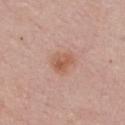Recorded during total-body skin imaging; not selected for excision or biopsy.
Approximately 3 mm at its widest.
A region of skin cropped from a whole-body photographic capture, roughly 15 mm wide.
A male subject, aged 58 to 62.
The lesion is located on the chest.
The lesion-visualizer software estimated a border-irregularity index near 2.5/10, internal color variation of about 4 on a 0–10 scale, and peripheral color asymmetry of about 1.5.
Imaged with white-light lighting.